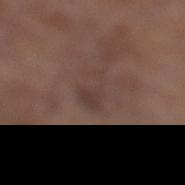Recorded during total-body skin imaging; not selected for excision or biopsy.
The patient is a male in their 80s.
From the left lower leg.
Longest diameter approximately 3.5 mm.
Cropped from a whole-body photographic skin survey; the tile spans about 15 mm.
Imaged with white-light lighting.
The total-body-photography lesion software estimated a border-irregularity index near 6.5/10, internal color variation of about 1.5 on a 0–10 scale, and radial color variation of about 0.5.